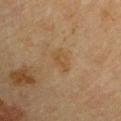{"biopsy_status": "not biopsied; imaged during a skin examination", "image": {"source": "total-body photography crop", "field_of_view_mm": 15}, "patient": {"sex": "male", "age_approx": 65}, "site": "right upper arm", "automated_metrics": {"area_mm2_approx": 3.5, "eccentricity": 0.8, "shape_asymmetry": 0.3, "nevus_likeness_0_100": 0, "lesion_detection_confidence_0_100": 100}, "lighting": "cross-polarized", "lesion_size": {"long_diameter_mm_approx": 3.0}}